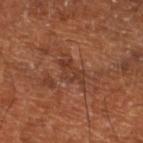<lesion>
<biopsy_status>not biopsied; imaged during a skin examination</biopsy_status>
<patient>
  <age_approx>65</age_approx>
</patient>
<lighting>cross-polarized</lighting>
<site>leg</site>
<image>
  <source>total-body photography crop</source>
  <field_of_view_mm>15</field_of_view_mm>
</image>
<lesion_size>
  <long_diameter_mm_approx>4.0</long_diameter_mm_approx>
</lesion_size>
</lesion>